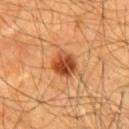Part of a total-body skin-imaging series; this lesion was reviewed on a skin check and was not flagged for biopsy.
On the chest.
The subject is a male roughly 60 years of age.
Cropped from a total-body skin-imaging series; the visible field is about 15 mm.
Automated image analysis of the tile measured a lesion area of about 7.5 mm², a shape eccentricity near 0.3, and a shape-asymmetry score of about 0.2 (0 = symmetric). The software also gave a within-lesion color-variation index near 6.5/10 and radial color variation of about 2. It also reported an automated nevus-likeness rating near 100 out of 100.
About 3 mm across.
This is a cross-polarized tile.